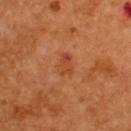| field | value |
|---|---|
| notes | imaged on a skin check; not biopsied |
| lesion diameter | ≈3 mm |
| illumination | cross-polarized |
| image-analysis metrics | a lesion color around L≈42 a*≈27 b*≈35 in CIELAB, a lesion–skin lightness drop of about 6, and a normalized border contrast of about 5 |
| subject | male, approximately 55 years of age |
| image source | total-body-photography crop, ~15 mm field of view |
| body site | the back |